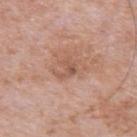follow-up — catalogued during a skin exam; not biopsied
image source — ~15 mm tile from a whole-body skin photo
illumination — white-light illumination
image-analysis metrics — an outline eccentricity of about 0.7 (0 = round, 1 = elongated); a classifier nevus-likeness of about 0/100 and a detector confidence of about 100 out of 100 that the crop contains a lesion
subject — male, in their 80s
location — the arm
size — about 2.5 mm